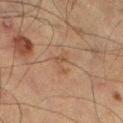No biopsy was performed on this lesion — it was imaged during a full skin examination and was not determined to be concerning.
From the left thigh.
Cropped from a total-body skin-imaging series; the visible field is about 15 mm.
A male subject aged 63 to 67.
The tile uses cross-polarized illumination.